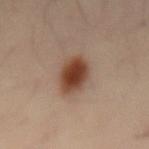Impression: Part of a total-body skin-imaging series; this lesion was reviewed on a skin check and was not flagged for biopsy. Acquisition and patient details: The lesion is located on the back. About 5 mm across. Imaged with cross-polarized lighting. A region of skin cropped from a whole-body photographic capture, roughly 15 mm wide. A male subject aged around 40.